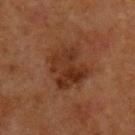Q: Was a biopsy performed?
A: catalogued during a skin exam; not biopsied
Q: Illumination type?
A: cross-polarized illumination
Q: What is the lesion's diameter?
A: ≈5 mm
Q: What kind of image is this?
A: total-body-photography crop, ~15 mm field of view
Q: What are the patient's age and sex?
A: male, in their mid-60s
Q: Lesion location?
A: the upper back